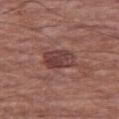Q: Was a biopsy performed?
A: catalogued during a skin exam; not biopsied
Q: Patient demographics?
A: male, about 65 years old
Q: What did automated image analysis measure?
A: an average lesion color of about L≈40 a*≈22 b*≈21 (CIELAB), roughly 10 lightness units darker than nearby skin, and a normalized border contrast of about 8; a detector confidence of about 100 out of 100 that the crop contains a lesion
Q: Illumination type?
A: white-light illumination
Q: What is the anatomic site?
A: the right thigh
Q: What kind of image is this?
A: ~15 mm tile from a whole-body skin photo